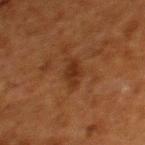Impression: No biopsy was performed on this lesion — it was imaged during a full skin examination and was not determined to be concerning. Image and clinical context: A 15 mm close-up extracted from a 3D total-body photography capture. This is a cross-polarized tile. The patient is a female aged around 50. From the upper back.